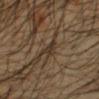Clinical impression: This lesion was catalogued during total-body skin photography and was not selected for biopsy. Acquisition and patient details: A region of skin cropped from a whole-body photographic capture, roughly 15 mm wide. The lesion is located on the left forearm. A male patient aged 43–47.